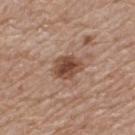A male subject, aged 78–82. Located on the upper back. The lesion-visualizer software estimated an area of roughly 7.5 mm² and a shape eccentricity near 0.65. The software also gave a lesion–skin lightness drop of about 13 and a lesion-to-skin contrast of about 9.5 (normalized; higher = more distinct). And it measured lesion-presence confidence of about 100/100. A 15 mm close-up tile from a total-body photography series done for melanoma screening.